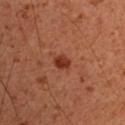Assessment:
Part of a total-body skin-imaging series; this lesion was reviewed on a skin check and was not flagged for biopsy.
Clinical summary:
Located on the chest. Captured under cross-polarized illumination. The subject is a male aged around 50. About 2 mm across. Cropped from a total-body skin-imaging series; the visible field is about 15 mm.